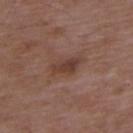The lesion was photographed on a routine skin check and not biopsied; there is no pathology result. The subject is a male aged around 50. A 15 mm crop from a total-body photograph taken for skin-cancer surveillance. Located on the upper back. The tile uses white-light illumination.